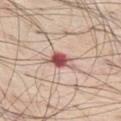Clinical impression:
Part of a total-body skin-imaging series; this lesion was reviewed on a skin check and was not flagged for biopsy.
Context:
A male subject, in their 70s. A lesion tile, about 15 mm wide, cut from a 3D total-body photograph. The lesion is located on the left thigh. Automated tile analysis of the lesion measured a lesion color around L≈56 a*≈26 b*≈24 in CIELAB and a lesion–skin lightness drop of about 16. Imaged with white-light lighting.